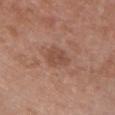Findings:
- biopsy status — no biopsy performed (imaged during a skin exam)
- site — the chest
- diameter — ≈3 mm
- tile lighting — white-light
- imaging modality — total-body-photography crop, ~15 mm field of view
- patient — female, roughly 40 years of age
- automated metrics — a footprint of about 6 mm² and a shape-asymmetry score of about 0.25 (0 = symmetric); a lesion color around L≈48 a*≈21 b*≈28 in CIELAB, roughly 8 lightness units darker than nearby skin, and a normalized border contrast of about 6; border irregularity of about 2 on a 0–10 scale and a peripheral color-asymmetry measure near 0.5; a classifier nevus-likeness of about 0/100 and lesion-presence confidence of about 100/100